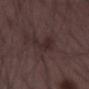<case>
<biopsy_status>not biopsied; imaged during a skin examination</biopsy_status>
<lighting>white-light</lighting>
<automated_metrics>
  <area_mm2_approx>6.0</area_mm2_approx>
  <shape_asymmetry>0.55</shape_asymmetry>
  <cielab_L>26</cielab_L>
  <cielab_a>15</cielab_a>
  <cielab_b>15</cielab_b>
  <color_variation_0_10>3.0</color_variation_0_10>
  <peripheral_color_asymmetry>1.0</peripheral_color_asymmetry>
  <nevus_likeness_0_100>0</nevus_likeness_0_100>
</automated_metrics>
<lesion_size>
  <long_diameter_mm_approx>3.5</long_diameter_mm_approx>
</lesion_size>
<site>left thigh</site>
<patient>
  <sex>male</sex>
  <age_approx>50</age_approx>
</patient>
<image>
  <source>total-body photography crop</source>
  <field_of_view_mm>15</field_of_view_mm>
</image>
</case>